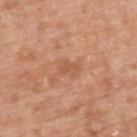The lesion was photographed on a routine skin check and not biopsied; there is no pathology result.
Approximately 2.5 mm at its widest.
The total-body-photography lesion software estimated a footprint of about 3 mm² and a shape-asymmetry score of about 0.35 (0 = symmetric). The analysis additionally found border irregularity of about 3.5 on a 0–10 scale, a color-variation rating of about 1/10, and a peripheral color-asymmetry measure near 0.5.
A male subject roughly 50 years of age.
Located on the upper back.
This is a white-light tile.
A 15 mm crop from a total-body photograph taken for skin-cancer surveillance.